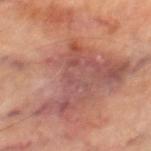Findings:
* workup: catalogued during a skin exam; not biopsied
* illumination: cross-polarized
* body site: the right thigh
* image source: ~15 mm tile from a whole-body skin photo
* patient: male, approximately 70 years of age
* TBP lesion metrics: border irregularity of about 9 on a 0–10 scale and internal color variation of about 8 on a 0–10 scale; a nevus-likeness score of about 20/100 and a lesion-detection confidence of about 80/100